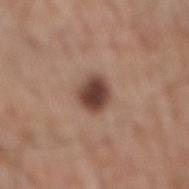Image and clinical context:
This image is a 15 mm lesion crop taken from a total-body photograph. The lesion is located on the mid back. A male subject in their 60s. Automated tile analysis of the lesion measured a lesion area of about 7.5 mm², an eccentricity of roughly 0.55, and two-axis asymmetry of about 0.15. The analysis additionally found a peripheral color-asymmetry measure near 1. And it measured lesion-presence confidence of about 100/100. Captured under white-light illumination.